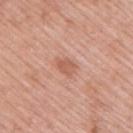Clinical impression:
The lesion was tiled from a total-body skin photograph and was not biopsied.
Context:
The lesion's longest dimension is about 2.5 mm. Captured under white-light illumination. A roughly 15 mm field-of-view crop from a total-body skin photograph. A male subject approximately 55 years of age. The lesion is located on the upper back. The lesion-visualizer software estimated a border-irregularity index near 2/10, a color-variation rating of about 1.5/10, and radial color variation of about 0.5.